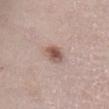| feature | finding |
|---|---|
| biopsy status | catalogued during a skin exam; not biopsied |
| patient | female, roughly 65 years of age |
| location | the abdomen |
| automated lesion analysis | a footprint of about 4.5 mm², an eccentricity of roughly 0.7, and a shape-asymmetry score of about 0.2 (0 = symmetric); an average lesion color of about L≈54 a*≈19 b*≈23 (CIELAB), a lesion–skin lightness drop of about 13, and a lesion-to-skin contrast of about 9 (normalized; higher = more distinct); a classifier nevus-likeness of about 90/100 |
| image source | ~15 mm tile from a whole-body skin photo |
| diameter | ~2.5 mm (longest diameter) |